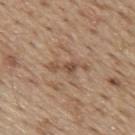No biopsy was performed on this lesion — it was imaged during a full skin examination and was not determined to be concerning.
Captured under white-light illumination.
A close-up tile cropped from a whole-body skin photograph, about 15 mm across.
A male patient, aged around 70.
The lesion is located on the mid back.